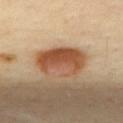Assessment: No biopsy was performed on this lesion — it was imaged during a full skin examination and was not determined to be concerning. Acquisition and patient details: The lesion is on the upper back. A female patient aged approximately 40. A region of skin cropped from a whole-body photographic capture, roughly 15 mm wide.